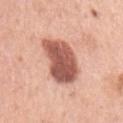Part of a total-body skin-imaging series; this lesion was reviewed on a skin check and was not flagged for biopsy.
The lesion's longest dimension is about 5 mm.
Imaged with white-light lighting.
A close-up tile cropped from a whole-body skin photograph, about 15 mm across.
The lesion is located on the left upper arm.
A female patient, about 60 years old.